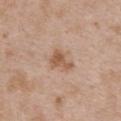Clinical impression: No biopsy was performed on this lesion — it was imaged during a full skin examination and was not determined to be concerning. Background: The patient is a female aged approximately 30. This is a white-light tile. From the upper back. Automated image analysis of the tile measured a mean CIELAB color near L≈56 a*≈19 b*≈31, a lesion–skin lightness drop of about 10, and a lesion-to-skin contrast of about 7.5 (normalized; higher = more distinct). The analysis additionally found a border-irregularity index near 4.5/10 and a within-lesion color-variation index near 2.5/10. The analysis additionally found a nevus-likeness score of about 15/100. Cropped from a whole-body photographic skin survey; the tile spans about 15 mm. About 3 mm across.